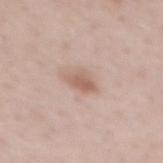| feature | finding |
|---|---|
| automated lesion analysis | an area of roughly 4.5 mm² and a shape-asymmetry score of about 0.25 (0 = symmetric); roughly 10 lightness units darker than nearby skin and a normalized border contrast of about 6.5; a classifier nevus-likeness of about 70/100 |
| patient | male, about 40 years old |
| site | the mid back |
| image source | 15 mm crop, total-body photography |
| lighting | white-light illumination |
| lesion size | ≈3 mm |